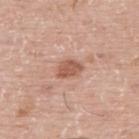The lesion was photographed on a routine skin check and not biopsied; there is no pathology result.
Located on the upper back.
This image is a 15 mm lesion crop taken from a total-body photograph.
Measured at roughly 3 mm in maximum diameter.
An algorithmic analysis of the crop reported an area of roughly 5 mm² and an outline eccentricity of about 0.75 (0 = round, 1 = elongated). It also reported a mean CIELAB color near L≈56 a*≈23 b*≈30, about 12 CIELAB-L* units darker than the surrounding skin, and a normalized lesion–skin contrast near 8.
The patient is a male in their mid- to late 70s.
Imaged with white-light lighting.